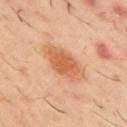A region of skin cropped from a whole-body photographic capture, roughly 15 mm wide. A male patient, approximately 60 years of age. Imaged with cross-polarized lighting. From the upper back. An algorithmic analysis of the crop reported an area of roughly 12 mm², a shape eccentricity near 0.85, and a shape-asymmetry score of about 0.15 (0 = symmetric). It also reported an average lesion color of about L≈57 a*≈24 b*≈36 (CIELAB), about 10 CIELAB-L* units darker than the surrounding skin, and a lesion-to-skin contrast of about 7.5 (normalized; higher = more distinct). It also reported a color-variation rating of about 3/10 and radial color variation of about 1. And it measured a classifier nevus-likeness of about 95/100.